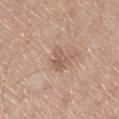* follow-up: total-body-photography surveillance lesion; no biopsy
* patient: female, approximately 55 years of age
* diameter: ≈2.5 mm
* illumination: white-light
* site: the left lower leg
* acquisition: total-body-photography crop, ~15 mm field of view